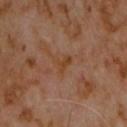biopsy status = total-body-photography surveillance lesion; no biopsy
site = the chest
TBP lesion metrics = a lesion area of about 3 mm²; a mean CIELAB color near L≈38 a*≈19 b*≈31, about 5 CIELAB-L* units darker than the surrounding skin, and a normalized border contrast of about 7; border irregularity of about 5 on a 0–10 scale and peripheral color asymmetry of about 0.5; an automated nevus-likeness rating near 0 out of 100 and lesion-presence confidence of about 100/100
illumination = cross-polarized
subject = male, in their 60s
image = ~15 mm crop, total-body skin-cancer survey